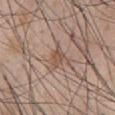{"biopsy_status": "not biopsied; imaged during a skin examination", "patient": {"sex": "male", "age_approx": 45}, "image": {"source": "total-body photography crop", "field_of_view_mm": 15}, "site": "abdomen", "lesion_size": {"long_diameter_mm_approx": 2.5}, "automated_metrics": {"shape_asymmetry": 0.25, "border_irregularity_0_10": 2.5, "peripheral_color_asymmetry": 0.5}}